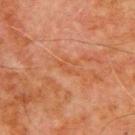Q: Is there a histopathology result?
A: no biopsy performed (imaged during a skin exam)
Q: What is the imaging modality?
A: ~15 mm crop, total-body skin-cancer survey
Q: Patient demographics?
A: male, in their 70s
Q: What is the lesion's diameter?
A: ≈3 mm
Q: Lesion location?
A: the chest